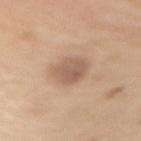Automated tile analysis of the lesion measured an eccentricity of roughly 0.35.
A male patient roughly 70 years of age.
A region of skin cropped from a whole-body photographic capture, roughly 15 mm wide.
The tile uses white-light illumination.
The lesion is located on the right upper arm.